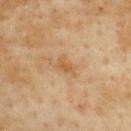Recorded during total-body skin imaging; not selected for excision or biopsy.
A female subject aged 58–62.
A close-up tile cropped from a whole-body skin photograph, about 15 mm across.
The lesion is located on the upper back.